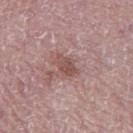Part of a total-body skin-imaging series; this lesion was reviewed on a skin check and was not flagged for biopsy. The subject is a male about 75 years old. From the left thigh. The total-body-photography lesion software estimated a lesion area of about 5 mm², an eccentricity of roughly 0.75, and two-axis asymmetry of about 0.3. The analysis additionally found roughly 9 lightness units darker than nearby skin and a normalized lesion–skin contrast near 6.5. And it measured a classifier nevus-likeness of about 0/100 and a lesion-detection confidence of about 100/100. Measured at roughly 3 mm in maximum diameter. A 15 mm close-up tile from a total-body photography series done for melanoma screening. Imaged with white-light lighting.